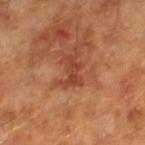Impression:
Part of a total-body skin-imaging series; this lesion was reviewed on a skin check and was not flagged for biopsy.
Context:
A male patient, in their mid- to late 60s. The tile uses cross-polarized illumination. A lesion tile, about 15 mm wide, cut from a 3D total-body photograph. The recorded lesion diameter is about 3.5 mm.